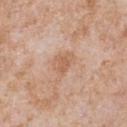Q: Was this lesion biopsied?
A: total-body-photography surveillance lesion; no biopsy
Q: Lesion location?
A: the front of the torso
Q: What is the imaging modality?
A: 15 mm crop, total-body photography
Q: What did automated image analysis measure?
A: a lesion color around L≈60 a*≈20 b*≈32 in CIELAB, roughly 8 lightness units darker than nearby skin, and a normalized border contrast of about 6; a border-irregularity rating of about 2/10, a within-lesion color-variation index near 1.5/10, and peripheral color asymmetry of about 0.5; a classifier nevus-likeness of about 0/100 and a detector confidence of about 100 out of 100 that the crop contains a lesion
Q: What are the patient's age and sex?
A: male, aged around 65
Q: What is the lesion's diameter?
A: about 3 mm